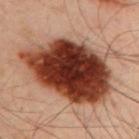Q: Is there a histopathology result?
A: no biopsy performed (imaged during a skin exam)
Q: Illumination type?
A: cross-polarized
Q: What is the lesion's diameter?
A: ~11 mm (longest diameter)
Q: What are the patient's age and sex?
A: male, in their 50s
Q: Lesion location?
A: the left arm
Q: What kind of image is this?
A: total-body-photography crop, ~15 mm field of view